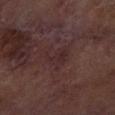biopsy status = catalogued during a skin exam; not biopsied | lesion diameter = ≈3 mm | imaging modality = total-body-photography crop, ~15 mm field of view | subject = male, roughly 70 years of age | tile lighting = cross-polarized | site = the right lower leg.